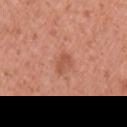Case summary:
– workup · total-body-photography surveillance lesion; no biopsy
– patient · female, approximately 35 years of age
– image source · 15 mm crop, total-body photography
– TBP lesion metrics · a border-irregularity index near 2.5/10, a within-lesion color-variation index near 1/10, and radial color variation of about 0.5
– tile lighting · white-light illumination
– body site · the left upper arm
– lesion diameter · ~2.5 mm (longest diameter)